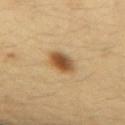notes: catalogued during a skin exam; not biopsied
image source: ~15 mm crop, total-body skin-cancer survey
illumination: cross-polarized
location: the left forearm
automated lesion analysis: a footprint of about 6.5 mm² and an eccentricity of roughly 0.6; a lesion color around L≈47 a*≈17 b*≈35 in CIELAB, roughly 14 lightness units darker than nearby skin, and a lesion-to-skin contrast of about 10 (normalized; higher = more distinct); a border-irregularity rating of about 1.5/10 and radial color variation of about 2; a classifier nevus-likeness of about 100/100 and lesion-presence confidence of about 100/100
patient: female, about 30 years old